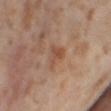Part of a total-body skin-imaging series; this lesion was reviewed on a skin check and was not flagged for biopsy.
Longest diameter approximately 3 mm.
A roughly 15 mm field-of-view crop from a total-body skin photograph.
From the leg.
A female patient, approximately 55 years of age.